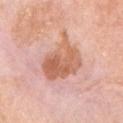Measured at roughly 6 mm in maximum diameter. A female patient roughly 75 years of age. This image is a 15 mm lesion crop taken from a total-body photograph. Imaged with white-light lighting. From the left upper arm.